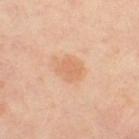The lesion was photographed on a routine skin check and not biopsied; there is no pathology result. The total-body-photography lesion software estimated an area of roughly 5 mm² and two-axis asymmetry of about 0.25. The software also gave lesion-presence confidence of about 100/100. A female subject, approximately 50 years of age. The lesion is on the left thigh. Imaged with cross-polarized lighting. A roughly 15 mm field-of-view crop from a total-body skin photograph.